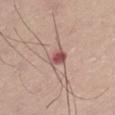Findings:
• biopsy status · no biopsy performed (imaged during a skin exam)
• imaging modality · ~15 mm tile from a whole-body skin photo
• subject · male, aged approximately 60
• location · the left thigh